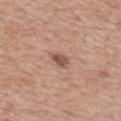{
  "biopsy_status": "not biopsied; imaged during a skin examination",
  "automated_metrics": {
    "cielab_L": 52,
    "cielab_a": 21,
    "cielab_b": 26,
    "vs_skin_darker_L": 12.0,
    "vs_skin_contrast_norm": 8.5,
    "nevus_likeness_0_100": 60,
    "lesion_detection_confidence_0_100": 100
  },
  "patient": {
    "sex": "male",
    "age_approx": 80
  },
  "image": {
    "source": "total-body photography crop",
    "field_of_view_mm": 15
  },
  "site": "mid back",
  "lighting": "white-light",
  "lesion_size": {
    "long_diameter_mm_approx": 2.5
  }
}